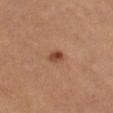Context:
A male patient, roughly 55 years of age. Cropped from a whole-body photographic skin survey; the tile spans about 15 mm. Automated tile analysis of the lesion measured an area of roughly 2.5 mm². From the left thigh. Imaged with cross-polarized lighting. Measured at roughly 2 mm in maximum diameter.